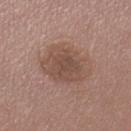workup — total-body-photography surveillance lesion; no biopsy | image-analysis metrics — border irregularity of about 2 on a 0–10 scale, internal color variation of about 3.5 on a 0–10 scale, and peripheral color asymmetry of about 1 | imaging modality — total-body-photography crop, ~15 mm field of view | location — the left upper arm | tile lighting — white-light | lesion diameter — about 6 mm | subject — female, about 50 years old.